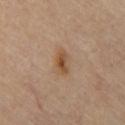Recorded during total-body skin imaging; not selected for excision or biopsy. The recorded lesion diameter is about 3.5 mm. A region of skin cropped from a whole-body photographic capture, roughly 15 mm wide. Automated tile analysis of the lesion measured an average lesion color of about L≈51 a*≈19 b*≈34 (CIELAB), about 9 CIELAB-L* units darker than the surrounding skin, and a normalized lesion–skin contrast near 8. The analysis additionally found border irregularity of about 2 on a 0–10 scale, a within-lesion color-variation index near 4.5/10, and radial color variation of about 1.5. From the chest. Captured under cross-polarized illumination.